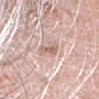Findings:
- notes · total-body-photography surveillance lesion; no biopsy
- subject · female, aged approximately 75
- imaging modality · ~15 mm tile from a whole-body skin photo
- anatomic site · the head or neck